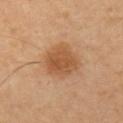Clinical impression:
Recorded during total-body skin imaging; not selected for excision or biopsy.
Image and clinical context:
From the left upper arm. A lesion tile, about 15 mm wide, cut from a 3D total-body photograph. The subject is a female aged 38 to 42.